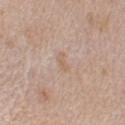<lesion>
  <biopsy_status>not biopsied; imaged during a skin examination</biopsy_status>
  <lighting>white-light</lighting>
  <site>left upper arm</site>
  <lesion_size>
    <long_diameter_mm_approx>2.5</long_diameter_mm_approx>
  </lesion_size>
  <image>
    <source>total-body photography crop</source>
    <field_of_view_mm>15</field_of_view_mm>
  </image>
  <patient>
    <sex>male</sex>
    <age_approx>45</age_approx>
  </patient>
  <automated_metrics>
    <color_variation_0_10>0.0</color_variation_0_10>
    <peripheral_color_asymmetry>0.0</peripheral_color_asymmetry>
    <lesion_detection_confidence_0_100>100</lesion_detection_confidence_0_100>
  </automated_metrics>
</lesion>